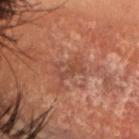biopsy status: imaged on a skin check; not biopsied
acquisition: ~15 mm tile from a whole-body skin photo
lesion diameter: about 3.5 mm
site: the head or neck
subject: female, in their mid- to late 50s